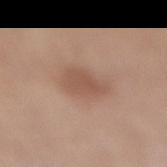Assessment: The lesion was tiled from a total-body skin photograph and was not biopsied. Acquisition and patient details: The subject is a female aged approximately 65. Measured at roughly 4 mm in maximum diameter. The lesion is located on the right lower leg. A 15 mm close-up extracted from a 3D total-body photography capture. Captured under white-light illumination.